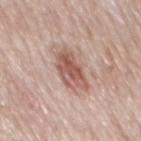The lesion was tiled from a total-body skin photograph and was not biopsied. A roughly 15 mm field-of-view crop from a total-body skin photograph. A male subject, about 75 years old. Automated image analysis of the tile measured border irregularity of about 3 on a 0–10 scale and a color-variation rating of about 6/10. It also reported a detector confidence of about 100 out of 100 that the crop contains a lesion. The lesion is on the mid back. The recorded lesion diameter is about 5 mm. Imaged with white-light lighting.